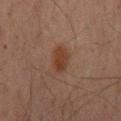follow-up: no biopsy performed (imaged during a skin exam) | patient: male, aged 68–72 | illumination: cross-polarized illumination | anatomic site: the abdomen | diameter: ~3.5 mm (longest diameter) | imaging modality: ~15 mm crop, total-body skin-cancer survey.